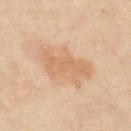The lesion was tiled from a total-body skin photograph and was not biopsied. Measured at roughly 6 mm in maximum diameter. A female subject aged approximately 50. Located on the left thigh. Automated tile analysis of the lesion measured a border-irregularity index near 5/10 and a peripheral color-asymmetry measure near 1. This image is a 15 mm lesion crop taken from a total-body photograph.